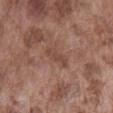workup: imaged on a skin check; not biopsied | tile lighting: white-light illumination | acquisition: ~15 mm tile from a whole-body skin photo | body site: the abdomen | patient: male, roughly 75 years of age | lesion size: about 3.5 mm | automated metrics: an average lesion color of about L≈45 a*≈20 b*≈26 (CIELAB) and a normalized border contrast of about 6; a border-irregularity rating of about 3.5/10 and peripheral color asymmetry of about 0; a nevus-likeness score of about 0/100 and lesion-presence confidence of about 100/100.